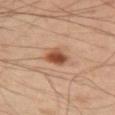patient = male, aged around 50 | body site = the left upper arm | image-analysis metrics = a lesion color around L≈49 a*≈23 b*≈33 in CIELAB, a lesion–skin lightness drop of about 14, and a normalized lesion–skin contrast near 10.5; a border-irregularity index near 2/10, a color-variation rating of about 4/10, and peripheral color asymmetry of about 1; a classifier nevus-likeness of about 95/100 and lesion-presence confidence of about 100/100 | tile lighting = cross-polarized | lesion size = ≈3 mm | imaging modality = ~15 mm crop, total-body skin-cancer survey.